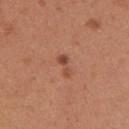<lesion>
<biopsy_status>not biopsied; imaged during a skin examination</biopsy_status>
<lighting>white-light</lighting>
<lesion_size>
  <long_diameter_mm_approx>3.0</long_diameter_mm_approx>
</lesion_size>
<site>right upper arm</site>
<image>
  <source>total-body photography crop</source>
  <field_of_view_mm>15</field_of_view_mm>
</image>
<patient>
  <sex>female</sex>
  <age_approx>30</age_approx>
</patient>
</lesion>